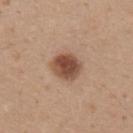Clinical impression:
Imaged during a routine full-body skin examination; the lesion was not biopsied and no histopathology is available.
Acquisition and patient details:
This is a white-light tile. Automated tile analysis of the lesion measured a footprint of about 9 mm², an outline eccentricity of about 0.5 (0 = round, 1 = elongated), and a symmetry-axis asymmetry near 0.15. It also reported a mean CIELAB color near L≈49 a*≈20 b*≈29 and a lesion-to-skin contrast of about 10.5 (normalized; higher = more distinct). Longest diameter approximately 3.5 mm. A male patient aged approximately 35. On the back. A 15 mm crop from a total-body photograph taken for skin-cancer surveillance.